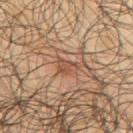Assessment: Recorded during total-body skin imaging; not selected for excision or biopsy. Background: Cropped from a whole-body photographic skin survey; the tile spans about 15 mm. Captured under cross-polarized illumination. The recorded lesion diameter is about 2.5 mm. The lesion is on the arm. A male patient, approximately 65 years of age.